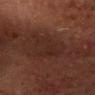Part of a total-body skin-imaging series; this lesion was reviewed on a skin check and was not flagged for biopsy.
A close-up tile cropped from a whole-body skin photograph, about 15 mm across.
The patient is a male approximately 65 years of age.
From the head or neck.